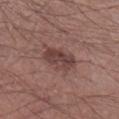This lesion was catalogued during total-body skin photography and was not selected for biopsy. The lesion is on the left lower leg. The subject is a male aged approximately 45. Cropped from a total-body skin-imaging series; the visible field is about 15 mm.